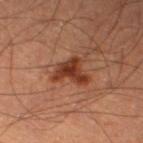workup: imaged on a skin check; not biopsied | imaging modality: 15 mm crop, total-body photography | lesion size: about 4.5 mm | anatomic site: the left thigh | subject: male, aged around 60 | illumination: cross-polarized.